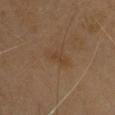This lesion was catalogued during total-body skin photography and was not selected for biopsy. Cropped from a total-body skin-imaging series; the visible field is about 15 mm. Captured under cross-polarized illumination. From the head or neck. A male patient, approximately 60 years of age. Approximately 3 mm at its widest.